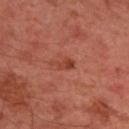  biopsy_status: not biopsied; imaged during a skin examination
  automated_metrics:
    eccentricity: 0.85
    shape_asymmetry: 0.45
  site: upper back
  image:
    source: total-body photography crop
    field_of_view_mm: 15
  patient:
    sex: male
    age_approx: 45
  lesion_size:
    long_diameter_mm_approx: 3.0
  lighting: cross-polarized